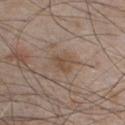Q: Is there a histopathology result?
A: no biopsy performed (imaged during a skin exam)
Q: How large is the lesion?
A: about 3 mm
Q: How was the tile lit?
A: white-light illumination
Q: Who is the patient?
A: male, aged approximately 50
Q: Automated lesion metrics?
A: a shape-asymmetry score of about 0.3 (0 = symmetric); a border-irregularity rating of about 2.5/10 and a peripheral color-asymmetry measure near 1
Q: What kind of image is this?
A: ~15 mm crop, total-body skin-cancer survey
Q: Lesion location?
A: the front of the torso